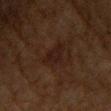Findings:
– notes: imaged on a skin check; not biopsied
– location: the left upper arm
– acquisition: ~15 mm crop, total-body skin-cancer survey
– subject: female, in their mid-50s
– lesion diameter: about 3 mm
– lighting: cross-polarized